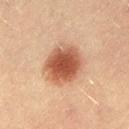{"biopsy_status": "not biopsied; imaged during a skin examination", "site": "lower back", "patient": {"sex": "female", "age_approx": 20}, "automated_metrics": {"area_mm2_approx": 18.0, "shape_asymmetry": 0.1}, "image": {"source": "total-body photography crop", "field_of_view_mm": 15}, "lesion_size": {"long_diameter_mm_approx": 5.0}, "lighting": "cross-polarized"}